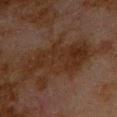Impression:
Recorded during total-body skin imaging; not selected for excision or biopsy.
Acquisition and patient details:
From the chest. Cropped from a total-body skin-imaging series; the visible field is about 15 mm. A male subject, roughly 80 years of age. Captured under cross-polarized illumination.